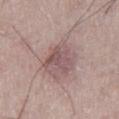Impression:
The lesion was tiled from a total-body skin photograph and was not biopsied.
Acquisition and patient details:
The lesion is located on the left thigh. Cropped from a total-body skin-imaging series; the visible field is about 15 mm. A male subject in their mid-70s.